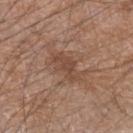Clinical impression: Imaged during a routine full-body skin examination; the lesion was not biopsied and no histopathology is available. Clinical summary: A male patient approximately 65 years of age. This image is a 15 mm lesion crop taken from a total-body photograph. This is a white-light tile. The lesion-visualizer software estimated a footprint of about 6.5 mm², an eccentricity of roughly 0.85, and two-axis asymmetry of about 0.35. The software also gave a lesion-to-skin contrast of about 6 (normalized; higher = more distinct). The lesion is on the arm.